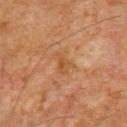{
  "biopsy_status": "not biopsied; imaged during a skin examination",
  "patient": {
    "sex": "male",
    "age_approx": 65
  },
  "lighting": "cross-polarized",
  "image": {
    "source": "total-body photography crop",
    "field_of_view_mm": 15
  },
  "site": "upper back",
  "automated_metrics": {
    "cielab_L": 41,
    "cielab_a": 19,
    "cielab_b": 32,
    "vs_skin_darker_L": 5.0,
    "vs_skin_contrast_norm": 5.5,
    "nevus_likeness_0_100": 0,
    "lesion_detection_confidence_0_100": 100
  },
  "lesion_size": {
    "long_diameter_mm_approx": 3.0
  }
}